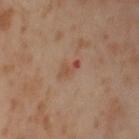Imaged during a routine full-body skin examination; the lesion was not biopsied and no histopathology is available. A lesion tile, about 15 mm wide, cut from a 3D total-body photograph. Located on the left thigh. The total-body-photography lesion software estimated a footprint of about 3.5 mm². And it measured a lesion color around L≈50 a*≈21 b*≈31 in CIELAB, about 7 CIELAB-L* units darker than the surrounding skin, and a normalized lesion–skin contrast near 6. It also reported a border-irregularity index near 3.5/10, a within-lesion color-variation index near 1/10, and peripheral color asymmetry of about 0.5. The analysis additionally found a nevus-likeness score of about 0/100 and a lesion-detection confidence of about 100/100. This is a cross-polarized tile. A female subject, aged 53–57. Longest diameter approximately 3 mm.